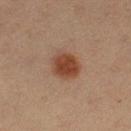Assessment: Recorded during total-body skin imaging; not selected for excision or biopsy. Background: A female subject aged approximately 20. The lesion is on the left thigh. This is a cross-polarized tile. Automated tile analysis of the lesion measured a lesion area of about 9 mm², an outline eccentricity of about 0.4 (0 = round, 1 = elongated), and a symmetry-axis asymmetry near 0.15. It also reported a mean CIELAB color near L≈37 a*≈20 b*≈27, roughly 10 lightness units darker than nearby skin, and a normalized border contrast of about 9.5. The analysis additionally found a border-irregularity rating of about 1/10, a color-variation rating of about 3/10, and a peripheral color-asymmetry measure near 1. The software also gave a lesion-detection confidence of about 100/100. Measured at roughly 3.5 mm in maximum diameter. A 15 mm close-up extracted from a 3D total-body photography capture.